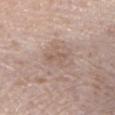Case summary:
* follow-up — total-body-photography surveillance lesion; no biopsy
* illumination — white-light
* anatomic site — the chest
* TBP lesion metrics — an average lesion color of about L≈57 a*≈16 b*≈25 (CIELAB), about 6 CIELAB-L* units darker than the surrounding skin, and a normalized border contrast of about 5; a border-irregularity index near 3/10, a within-lesion color-variation index near 0/10, and radial color variation of about 0
* image — total-body-photography crop, ~15 mm field of view
* lesion size — about 3 mm
* subject — female, roughly 45 years of age